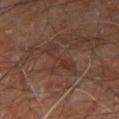{
  "biopsy_status": "not biopsied; imaged during a skin examination",
  "patient": {
    "sex": "male",
    "age_approx": 60
  },
  "image": {
    "source": "total-body photography crop",
    "field_of_view_mm": 15
  },
  "lesion_size": {
    "long_diameter_mm_approx": 5.5
  },
  "automated_metrics": {
    "cielab_L": 32,
    "cielab_a": 18,
    "cielab_b": 23,
    "vs_skin_darker_L": 5.0,
    "nevus_likeness_0_100": 0
  },
  "site": "leg",
  "lighting": "cross-polarized"
}